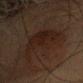{
  "biopsy_status": "not biopsied; imaged during a skin examination",
  "lesion_size": {
    "long_diameter_mm_approx": 9.0
  },
  "lighting": "cross-polarized",
  "patient": {
    "sex": "female",
    "age_approx": 65
  },
  "automated_metrics": {
    "nevus_likeness_0_100": 10,
    "lesion_detection_confidence_0_100": 50
  },
  "site": "head or neck",
  "image": {
    "source": "total-body photography crop",
    "field_of_view_mm": 15
  }
}